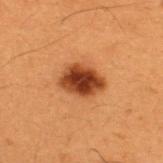<tbp_lesion>
  <biopsy_status>not biopsied; imaged during a skin examination</biopsy_status>
  <lighting>cross-polarized</lighting>
  <image>
    <source>total-body photography crop</source>
    <field_of_view_mm>15</field_of_view_mm>
  </image>
  <patient>
    <sex>male</sex>
    <age_approx>50</age_approx>
  </patient>
  <site>upper back</site>
  <lesion_size>
    <long_diameter_mm_approx>4.5</long_diameter_mm_approx>
  </lesion_size>
  <automated_metrics>
    <area_mm2_approx>11.0</area_mm2_approx>
    <eccentricity>0.65</eccentricity>
    <shape_asymmetry>0.15</shape_asymmetry>
    <border_irregularity_0_10>1.5</border_irregularity_0_10>
    <color_variation_0_10>5.0</color_variation_0_10>
    <peripheral_color_asymmetry>1.5</peripheral_color_asymmetry>
  </automated_metrics>
</tbp_lesion>